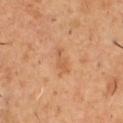illumination — cross-polarized; diameter — ~3.5 mm (longest diameter); location — the front of the torso; patient — male, about 50 years old; image — total-body-photography crop, ~15 mm field of view.